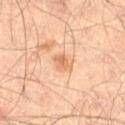Q: Was this lesion biopsied?
A: total-body-photography surveillance lesion; no biopsy
Q: Lesion location?
A: the right thigh
Q: What is the lesion's diameter?
A: about 2.5 mm
Q: How was the tile lit?
A: cross-polarized
Q: Automated lesion metrics?
A: border irregularity of about 3 on a 0–10 scale, internal color variation of about 2.5 on a 0–10 scale, and a peripheral color-asymmetry measure near 1
Q: What kind of image is this?
A: total-body-photography crop, ~15 mm field of view
Q: What are the patient's age and sex?
A: male, roughly 65 years of age